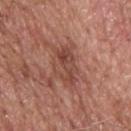– workup: imaged on a skin check; not biopsied
– diameter: ≈5 mm
– image source: ~15 mm tile from a whole-body skin photo
– patient: male, approximately 60 years of age
– automated metrics: a within-lesion color-variation index near 5.5/10 and peripheral color asymmetry of about 2; a classifier nevus-likeness of about 0/100 and a detector confidence of about 100 out of 100 that the crop contains a lesion
– body site: the upper back